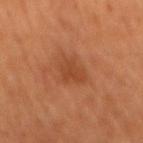Impression: Part of a total-body skin-imaging series; this lesion was reviewed on a skin check and was not flagged for biopsy. Context: A lesion tile, about 15 mm wide, cut from a 3D total-body photograph. A male patient aged around 65. The lesion is located on the mid back. This is a cross-polarized tile. The recorded lesion diameter is about 3 mm.